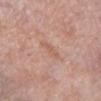Part of a total-body skin-imaging series; this lesion was reviewed on a skin check and was not flagged for biopsy.
From the left lower leg.
A male subject in their mid-70s.
Cropped from a total-body skin-imaging series; the visible field is about 15 mm.